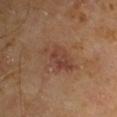– notes · catalogued during a skin exam; not biopsied
– site · the upper back
– illumination · cross-polarized
– image source · ~15 mm crop, total-body skin-cancer survey
– patient · male, roughly 60 years of age